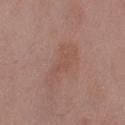Findings:
• biopsy status: no biopsy performed (imaged during a skin exam)
• patient: male, aged approximately 50
• anatomic site: the left upper arm
• illumination: white-light
• image: ~15 mm tile from a whole-body skin photo
• size: about 5.5 mm